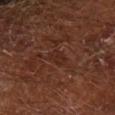Captured under cross-polarized illumination.
A 15 mm close-up tile from a total-body photography series done for melanoma screening.
Approximately 2.5 mm at its widest.
The subject is a male aged around 65.